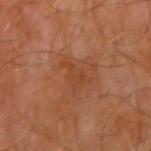Q: What kind of image is this?
A: total-body-photography crop, ~15 mm field of view
Q: Patient demographics?
A: male, roughly 65 years of age
Q: Lesion location?
A: the right upper arm